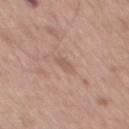{"biopsy_status": "not biopsied; imaged during a skin examination"}